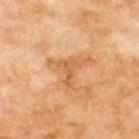workup = no biopsy performed (imaged during a skin exam)
patient = male, in their 70s
body site = the upper back
tile lighting = cross-polarized illumination
image = ~15 mm crop, total-body skin-cancer survey
automated lesion analysis = an average lesion color of about L≈61 a*≈24 b*≈43 (CIELAB), a lesion–skin lightness drop of about 8, and a normalized border contrast of about 6; an automated nevus-likeness rating near 0 out of 100 and a lesion-detection confidence of about 100/100
diameter = ≈5 mm